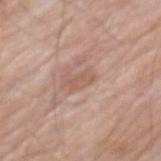Clinical impression: The lesion was tiled from a total-body skin photograph and was not biopsied. Image and clinical context: Cropped from a whole-body photographic skin survey; the tile spans about 15 mm. The subject is a male aged around 80. The lesion is located on the mid back.